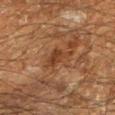Clinical summary: The lesion's longest dimension is about 3.5 mm. A 15 mm close-up extracted from a 3D total-body photography capture. The lesion is located on the left lower leg. Automated tile analysis of the lesion measured an eccentricity of roughly 0.8 and a shape-asymmetry score of about 0.4 (0 = symmetric). It also reported a within-lesion color-variation index near 1.5/10 and radial color variation of about 0.5. The patient is a male aged around 60.